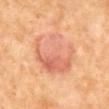Notes:
– notes · catalogued during a skin exam; not biopsied
– automated metrics · a within-lesion color-variation index near 7/10 and peripheral color asymmetry of about 2.5; a nevus-likeness score of about 40/100 and a detector confidence of about 100 out of 100 that the crop contains a lesion
– patient · female, about 50 years old
– anatomic site · the back
– tile lighting · cross-polarized illumination
– image source · total-body-photography crop, ~15 mm field of view